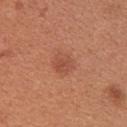The lesion was photographed on a routine skin check and not biopsied; there is no pathology result. An algorithmic analysis of the crop reported a mean CIELAB color near L≈50 a*≈27 b*≈33 and a normalized border contrast of about 5.5. The software also gave border irregularity of about 2 on a 0–10 scale. It also reported an automated nevus-likeness rating near 45 out of 100 and lesion-presence confidence of about 100/100. The patient is a female aged around 25. A 15 mm crop from a total-body photograph taken for skin-cancer surveillance. Located on the upper back. Longest diameter approximately 2.5 mm.